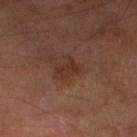Q: Was this lesion biopsied?
A: catalogued during a skin exam; not biopsied
Q: Lesion size?
A: ≈2.5 mm
Q: Automated lesion metrics?
A: border irregularity of about 4.5 on a 0–10 scale, internal color variation of about 2 on a 0–10 scale, and peripheral color asymmetry of about 0.5; a nevus-likeness score of about 10/100 and lesion-presence confidence of about 100/100
Q: Where on the body is the lesion?
A: the left lower leg
Q: What lighting was used for the tile?
A: cross-polarized illumination
Q: How was this image acquired?
A: ~15 mm tile from a whole-body skin photo
Q: Who is the patient?
A: male, aged 63–67